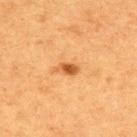biopsy status: total-body-photography surveillance lesion; no biopsy | image source: total-body-photography crop, ~15 mm field of view | TBP lesion metrics: a footprint of about 3 mm², an outline eccentricity of about 0.85 (0 = round, 1 = elongated), and a shape-asymmetry score of about 0.3 (0 = symmetric); about 13 CIELAB-L* units darker than the surrounding skin and a lesion-to-skin contrast of about 9 (normalized; higher = more distinct) | size: ≈2.5 mm | lighting: cross-polarized | patient: female, approximately 40 years of age | body site: the upper back.